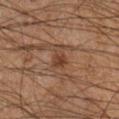| feature | finding |
|---|---|
| follow-up | no biopsy performed (imaged during a skin exam) |
| patient | male, approximately 60 years of age |
| acquisition | ~15 mm crop, total-body skin-cancer survey |
| anatomic site | the right lower leg |